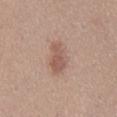Impression: Part of a total-body skin-imaging series; this lesion was reviewed on a skin check and was not flagged for biopsy. Acquisition and patient details: A region of skin cropped from a whole-body photographic capture, roughly 15 mm wide. A female patient, roughly 40 years of age. From the mid back.